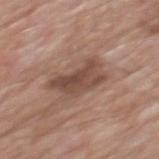This lesion was catalogued during total-body skin photography and was not selected for biopsy. A close-up tile cropped from a whole-body skin photograph, about 15 mm across. This is a white-light tile. Approximately 6 mm at its widest. From the mid back. A male subject, aged around 80.